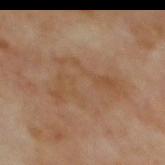Clinical impression:
Recorded during total-body skin imaging; not selected for excision or biopsy.
Background:
The total-body-photography lesion software estimated a shape-asymmetry score of about 0.7 (0 = symmetric). It also reported a border-irregularity index near 10/10 and peripheral color asymmetry of about 1. The lesion is on the mid back. A male patient aged 68 to 72. Approximately 7 mm at its widest. A roughly 15 mm field-of-view crop from a total-body skin photograph. The tile uses cross-polarized illumination.